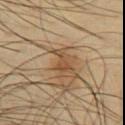biopsy status: no biopsy performed (imaged during a skin exam)
subject: male, aged 43–47
tile lighting: cross-polarized
image source: ~15 mm tile from a whole-body skin photo
size: about 3 mm
automated metrics: a footprint of about 3 mm² and an outline eccentricity of about 0.85 (0 = round, 1 = elongated); a nevus-likeness score of about 80/100 and a lesion-detection confidence of about 100/100
body site: the chest